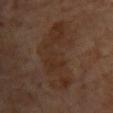The lesion was photographed on a routine skin check and not biopsied; there is no pathology result. Located on the upper back. Cropped from a whole-body photographic skin survey; the tile spans about 15 mm. A female patient in their mid-60s. An algorithmic analysis of the crop reported a lesion area of about 30 mm², a shape eccentricity near 0.8, and a shape-asymmetry score of about 0.4 (0 = symmetric). The software also gave internal color variation of about 3.5 on a 0–10 scale and peripheral color asymmetry of about 1. Approximately 8.5 mm at its widest.